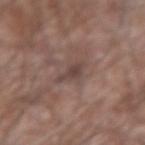No biopsy was performed on this lesion — it was imaged during a full skin examination and was not determined to be concerning.
The tile uses white-light illumination.
This image is a 15 mm lesion crop taken from a total-body photograph.
A male patient, aged around 60.
The recorded lesion diameter is about 3.5 mm.
From the left forearm.